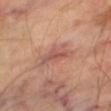Assessment: No biopsy was performed on this lesion — it was imaged during a full skin examination and was not determined to be concerning. Background: A region of skin cropped from a whole-body photographic capture, roughly 15 mm wide. A male patient aged 68–72. The lesion is on the right thigh.